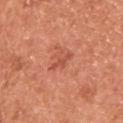Impression:
This lesion was catalogued during total-body skin photography and was not selected for biopsy.
Clinical summary:
A male subject, aged 53–57. From the upper back. Automated image analysis of the tile measured a mean CIELAB color near L≈53 a*≈32 b*≈35, about 8 CIELAB-L* units darker than the surrounding skin, and a lesion-to-skin contrast of about 6 (normalized; higher = more distinct). Longest diameter approximately 2.5 mm. A 15 mm close-up extracted from a 3D total-body photography capture.